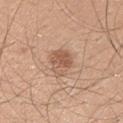Recorded during total-body skin imaging; not selected for excision or biopsy.
Located on the left upper arm.
Automated image analysis of the tile measured a lesion-to-skin contrast of about 7 (normalized; higher = more distinct).
The tile uses white-light illumination.
About 3.5 mm across.
A close-up tile cropped from a whole-body skin photograph, about 15 mm across.
A male subject, about 25 years old.